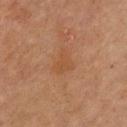Imaged with cross-polarized lighting.
Approximately 3 mm at its widest.
Located on the chest.
The subject is a male aged 43 to 47.
A 15 mm close-up extracted from a 3D total-body photography capture.